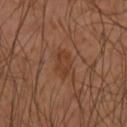notes = imaged on a skin check; not biopsied | image = 15 mm crop, total-body photography | location = the right forearm | lesion diameter = about 3.5 mm.